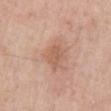The lesion was photographed on a routine skin check and not biopsied; there is no pathology result. Longest diameter approximately 3 mm. Cropped from a whole-body photographic skin survey; the tile spans about 15 mm. A male subject approximately 75 years of age. The lesion is located on the abdomen. Automated image analysis of the tile measured an area of roughly 5 mm², an eccentricity of roughly 0.7, and a symmetry-axis asymmetry near 0.2. It also reported a normalized border contrast of about 5.5. The software also gave a classifier nevus-likeness of about 0/100 and a detector confidence of about 100 out of 100 that the crop contains a lesion.